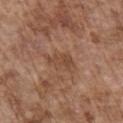• follow-up — imaged on a skin check; not biopsied
• image — total-body-photography crop, ~15 mm field of view
• subject — male, in their mid-70s
• tile lighting — white-light
• location — the front of the torso